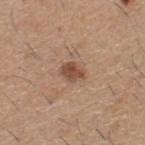– follow-up: total-body-photography surveillance lesion; no biopsy
– subject: male, approximately 60 years of age
– image: ~15 mm tile from a whole-body skin photo
– tile lighting: white-light illumination
– TBP lesion metrics: a footprint of about 4.5 mm², an outline eccentricity of about 0.65 (0 = round, 1 = elongated), and two-axis asymmetry of about 0.2; an average lesion color of about L≈49 a*≈21 b*≈30 (CIELAB) and a normalized border contrast of about 8; border irregularity of about 2 on a 0–10 scale and internal color variation of about 3 on a 0–10 scale; a nevus-likeness score of about 80/100 and a detector confidence of about 100 out of 100 that the crop contains a lesion
– anatomic site: the upper back
– size: about 3 mm